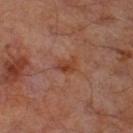Assessment: Imaged during a routine full-body skin examination; the lesion was not biopsied and no histopathology is available.